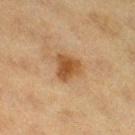Captured during whole-body skin photography for melanoma surveillance; the lesion was not biopsied.
Approximately 3.5 mm at its widest.
A female patient, aged 38–42.
From the right thigh.
The total-body-photography lesion software estimated an area of roughly 7 mm², a shape eccentricity near 0.35, and a symmetry-axis asymmetry near 0.35. The analysis additionally found roughly 10 lightness units darker than nearby skin. The analysis additionally found an automated nevus-likeness rating near 95 out of 100 and lesion-presence confidence of about 100/100.
A close-up tile cropped from a whole-body skin photograph, about 15 mm across.